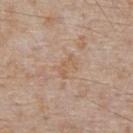workup: no biopsy performed (imaged during a skin exam); patient: male, aged around 65; site: the chest; lesion diameter: ≈3 mm; tile lighting: white-light illumination; image: 15 mm crop, total-body photography.